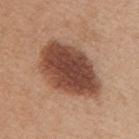<record>
  <biopsy_status>not biopsied; imaged during a skin examination</biopsy_status>
  <image>
    <source>total-body photography crop</source>
    <field_of_view_mm>15</field_of_view_mm>
  </image>
  <patient>
    <sex>female</sex>
    <age_approx>40</age_approx>
  </patient>
  <lesion_size>
    <long_diameter_mm_approx>8.0</long_diameter_mm_approx>
  </lesion_size>
  <lighting>white-light</lighting>
  <site>mid back</site>
</record>